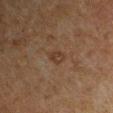Captured during whole-body skin photography for melanoma surveillance; the lesion was not biopsied. Automated image analysis of the tile measured an eccentricity of roughly 0.7 and a symmetry-axis asymmetry near 0.25. This is a cross-polarized tile. The subject is a male in their 60s. A 15 mm close-up tile from a total-body photography series done for melanoma screening. From the left upper arm.